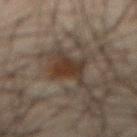| key | value |
|---|---|
| notes | catalogued during a skin exam; not biopsied |
| acquisition | ~15 mm crop, total-body skin-cancer survey |
| location | the abdomen |
| subject | male, aged around 65 |